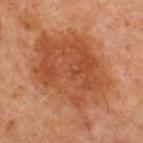Part of a total-body skin-imaging series; this lesion was reviewed on a skin check and was not flagged for biopsy. The lesion's longest dimension is about 11.5 mm. Located on the chest. Captured under cross-polarized illumination. The patient is a male about 65 years old. A region of skin cropped from a whole-body photographic capture, roughly 15 mm wide.